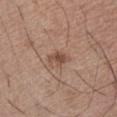Notes:
– follow-up: no biopsy performed (imaged during a skin exam)
– patient: female, aged approximately 75
– location: the abdomen
– lighting: white-light
– acquisition: total-body-photography crop, ~15 mm field of view